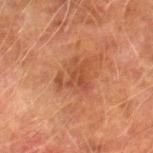This lesion was catalogued during total-body skin photography and was not selected for biopsy. Approximately 4.5 mm at its widest. An algorithmic analysis of the crop reported a footprint of about 9.5 mm², a shape eccentricity near 0.6, and a symmetry-axis asymmetry near 0.45. And it measured a lesion–skin lightness drop of about 7 and a lesion-to-skin contrast of about 6 (normalized; higher = more distinct). The analysis additionally found an automated nevus-likeness rating near 0 out of 100 and a detector confidence of about 100 out of 100 that the crop contains a lesion. Located on the leg. A male subject aged approximately 75. The tile uses cross-polarized illumination. A 15 mm close-up extracted from a 3D total-body photography capture.